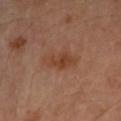anatomic site: the left forearm | subject: female, in their mid-60s | lighting: cross-polarized illumination | image: 15 mm crop, total-body photography | image-analysis metrics: a lesion area of about 7 mm², an eccentricity of roughly 0.8, and a shape-asymmetry score of about 0.2 (0 = symmetric); a mean CIELAB color near L≈43 a*≈22 b*≈31, about 7 CIELAB-L* units darker than the surrounding skin, and a lesion-to-skin contrast of about 7 (normalized; higher = more distinct); a classifier nevus-likeness of about 50/100 and lesion-presence confidence of about 100/100.